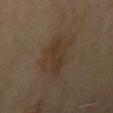biopsy status: no biopsy performed (imaged during a skin exam) | automated lesion analysis: a lesion color around L≈33 a*≈12 b*≈25 in CIELAB and roughly 5 lightness units darker than nearby skin | imaging modality: ~15 mm crop, total-body skin-cancer survey | body site: the right upper arm | tile lighting: cross-polarized illumination | lesion size: ≈4.5 mm | patient: male, in their mid-80s or older.